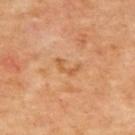Imaged during a routine full-body skin examination; the lesion was not biopsied and no histopathology is available.